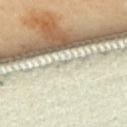Imaged during a routine full-body skin examination; the lesion was not biopsied and no histopathology is available. A female subject, aged 58–62. The lesion's longest dimension is about 1 mm. Automated image analysis of the tile measured a lesion area of about 0.5 mm², an eccentricity of roughly 0.85, and a symmetry-axis asymmetry near 0.55. The analysis additionally found a lesion color around L≈73 a*≈1 b*≈24 in CIELAB and a lesion-to-skin contrast of about 4 (normalized; higher = more distinct). The software also gave a border-irregularity rating of about 4.5/10, a color-variation rating of about 0/10, and a peripheral color-asymmetry measure near 0. From the upper back. A region of skin cropped from a whole-body photographic capture, roughly 15 mm wide.